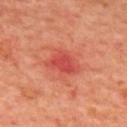Captured during whole-body skin photography for melanoma surveillance; the lesion was not biopsied.
The lesion is located on the upper back.
A male subject in their mid-60s.
A 15 mm close-up tile from a total-body photography series done for melanoma screening.